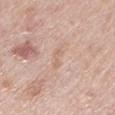No biopsy was performed on this lesion — it was imaged during a full skin examination and was not determined to be concerning.
Measured at roughly 3 mm in maximum diameter.
Located on the left lower leg.
A 15 mm close-up tile from a total-body photography series done for melanoma screening.
The tile uses white-light illumination.
The patient is a female approximately 65 years of age.